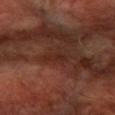Q: Where on the body is the lesion?
A: the right forearm
Q: What lighting was used for the tile?
A: cross-polarized
Q: Who is the patient?
A: aged approximately 65
Q: Lesion size?
A: ~2.5 mm (longest diameter)
Q: How was this image acquired?
A: total-body-photography crop, ~15 mm field of view